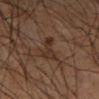<lesion>
<biopsy_status>not biopsied; imaged during a skin examination</biopsy_status>
</lesion>